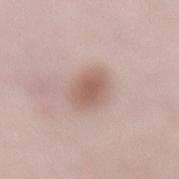Captured during whole-body skin photography for melanoma surveillance; the lesion was not biopsied.